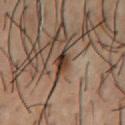notes: imaged on a skin check; not biopsied
automated metrics: a border-irregularity index near 2.5/10 and a peripheral color-asymmetry measure near 2; an automated nevus-likeness rating near 95 out of 100
lesion diameter: ~3 mm (longest diameter)
image source: ~15 mm tile from a whole-body skin photo
illumination: cross-polarized
location: the chest
subject: male, aged 53 to 57